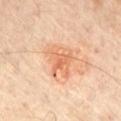{
  "biopsy_status": "not biopsied; imaged during a skin examination",
  "image": {
    "source": "total-body photography crop",
    "field_of_view_mm": 15
  },
  "lesion_size": {
    "long_diameter_mm_approx": 4.0
  },
  "site": "right thigh",
  "patient": {
    "sex": "male",
    "age_approx": 70
  },
  "automated_metrics": {
    "area_mm2_approx": 10.0,
    "eccentricity": 0.5,
    "shape_asymmetry": 0.4,
    "lesion_detection_confidence_0_100": 100
  }
}